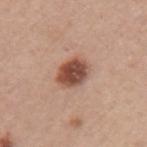Background:
On the left upper arm. The recorded lesion diameter is about 3.5 mm. A female patient, in their 60s. A region of skin cropped from a whole-body photographic capture, roughly 15 mm wide.